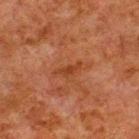| field | value |
|---|---|
| biopsy status | no biopsy performed (imaged during a skin exam) |
| tile lighting | cross-polarized |
| subject | male, approximately 80 years of age |
| location | the upper back |
| image | 15 mm crop, total-body photography |
| lesion diameter | ~3 mm (longest diameter) |